The lesion is located on the front of the torso · a region of skin cropped from a whole-body photographic capture, roughly 15 mm wide · the subject is a female in their 30s: 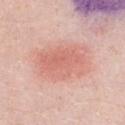Diagnosis: Biopsy histopathology demonstrated a dysplastic (Clark) nevus.